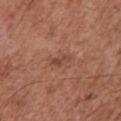Q: Is there a histopathology result?
A: total-body-photography surveillance lesion; no biopsy
Q: Where on the body is the lesion?
A: the chest
Q: What lighting was used for the tile?
A: white-light
Q: What is the imaging modality?
A: total-body-photography crop, ~15 mm field of view
Q: What are the patient's age and sex?
A: male, aged 73 to 77
Q: What is the lesion's diameter?
A: ~2.5 mm (longest diameter)